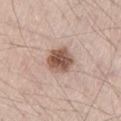The lesion was photographed on a routine skin check and not biopsied; there is no pathology result. Longest diameter approximately 4 mm. From the left thigh. A male subject, aged around 40. Cropped from a total-body skin-imaging series; the visible field is about 15 mm.